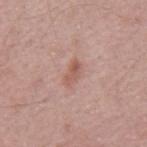| feature | finding |
|---|---|
| workup | catalogued during a skin exam; not biopsied |
| patient | male, aged approximately 65 |
| image | ~15 mm crop, total-body skin-cancer survey |
| diameter | about 3 mm |
| illumination | white-light |
| site | the back |